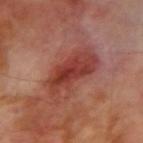Impression:
No biopsy was performed on this lesion — it was imaged during a full skin examination and was not determined to be concerning.
Image and clinical context:
From the arm. A male patient roughly 70 years of age. Approximately 6.5 mm at its widest. Imaged with cross-polarized lighting. Cropped from a total-body skin-imaging series; the visible field is about 15 mm.